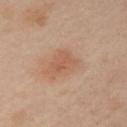No biopsy was performed on this lesion — it was imaged during a full skin examination and was not determined to be concerning. A close-up tile cropped from a whole-body skin photograph, about 15 mm across. A female subject about 40 years old. Captured under cross-polarized illumination. From the arm.